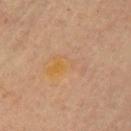Findings:
- notes — catalogued during a skin exam; not biopsied
- image source — total-body-photography crop, ~15 mm field of view
- image-analysis metrics — a mean CIELAB color near L≈52 a*≈16 b*≈34, a lesion–skin lightness drop of about 3, and a normalized lesion–skin contrast near 5; a nevus-likeness score of about 0/100 and a lesion-detection confidence of about 100/100
- patient — female, in their 60s
- site — the left upper arm
- lighting — cross-polarized illumination
- lesion size — ≈5 mm